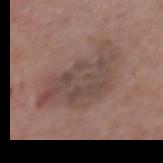biopsy status: catalogued during a skin exam; not biopsied | imaging modality: ~15 mm crop, total-body skin-cancer survey | subject: male, about 70 years old | site: the mid back.